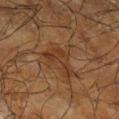Recorded during total-body skin imaging; not selected for excision or biopsy. The lesion is located on the right lower leg. A roughly 15 mm field-of-view crop from a total-body skin photograph. This is a cross-polarized tile. The patient is a male aged approximately 65.